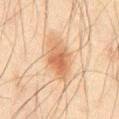The lesion was photographed on a routine skin check and not biopsied; there is no pathology result. This is a cross-polarized tile. A male patient about 45 years old. The lesion is on the abdomen. A lesion tile, about 15 mm wide, cut from a 3D total-body photograph.